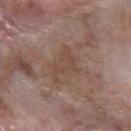The lesion was photographed on a routine skin check and not biopsied; there is no pathology result. A male subject in their 60s. Imaged with white-light lighting. On the left forearm. A 15 mm close-up extracted from a 3D total-body photography capture. About 5 mm across.